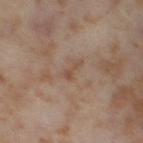notes: no biopsy performed (imaged during a skin exam) | image: ~15 mm crop, total-body skin-cancer survey | diameter: ≈2.5 mm | site: the right thigh | tile lighting: cross-polarized illumination | patient: female, in their mid-50s.